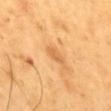The lesion was tiled from a total-body skin photograph and was not biopsied. A 15 mm crop from a total-body photograph taken for skin-cancer surveillance. The lesion is on the mid back. Captured under cross-polarized illumination. Automated tile analysis of the lesion measured a lesion–skin lightness drop of about 9 and a lesion-to-skin contrast of about 5.5 (normalized; higher = more distinct). The software also gave a border-irregularity rating of about 2/10 and internal color variation of about 0.5 on a 0–10 scale. And it measured a nevus-likeness score of about 5/100 and lesion-presence confidence of about 100/100. About 2.5 mm across. The patient is a male about 60 years old.